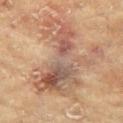The lesion is located on the arm.
About 9.5 mm across.
A close-up tile cropped from a whole-body skin photograph, about 15 mm across.
The lesion-visualizer software estimated two-axis asymmetry of about 0.55. The analysis additionally found about 9 CIELAB-L* units darker than the surrounding skin and a normalized lesion–skin contrast near 7. The software also gave border irregularity of about 9 on a 0–10 scale, internal color variation of about 9 on a 0–10 scale, and peripheral color asymmetry of about 2.5. The analysis additionally found lesion-presence confidence of about 95/100.
A female patient aged 78 to 82.